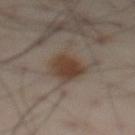Assessment: Imaged during a routine full-body skin examination; the lesion was not biopsied and no histopathology is available. Clinical summary: From the abdomen. Automated image analysis of the tile measured an outline eccentricity of about 0.55 (0 = round, 1 = elongated) and a shape-asymmetry score of about 0.2 (0 = symmetric). The analysis additionally found an average lesion color of about L≈40 a*≈14 b*≈24 (CIELAB) and a normalized border contrast of about 9.5. It also reported peripheral color asymmetry of about 1. And it measured a classifier nevus-likeness of about 95/100 and lesion-presence confidence of about 100/100. A roughly 15 mm field-of-view crop from a total-body skin photograph. A male patient in their mid-50s. Imaged with cross-polarized lighting. The recorded lesion diameter is about 4 mm.